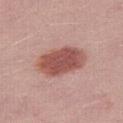Assessment:
Recorded during total-body skin imaging; not selected for excision or biopsy.
Image and clinical context:
This image is a 15 mm lesion crop taken from a total-body photograph. The lesion is located on the right thigh. The subject is a female aged around 25. Automated tile analysis of the lesion measured a lesion color around L≈52 a*≈25 b*≈25 in CIELAB, a lesion–skin lightness drop of about 14, and a normalized lesion–skin contrast near 9.5. The analysis additionally found border irregularity of about 1.5 on a 0–10 scale, internal color variation of about 3.5 on a 0–10 scale, and radial color variation of about 1. This is a white-light tile. About 5.5 mm across.